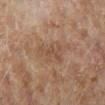Q: Was a biopsy performed?
A: total-body-photography surveillance lesion; no biopsy
Q: What is the anatomic site?
A: the right lower leg
Q: Lesion size?
A: ~4 mm (longest diameter)
Q: What did automated image analysis measure?
A: an area of roughly 6 mm², an outline eccentricity of about 0.85 (0 = round, 1 = elongated), and a symmetry-axis asymmetry near 0.55; a classifier nevus-likeness of about 0/100
Q: How was the tile lit?
A: cross-polarized
Q: What kind of image is this?
A: ~15 mm crop, total-body skin-cancer survey
Q: Who is the patient?
A: female, roughly 60 years of age